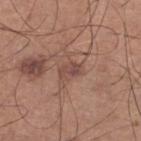Captured during whole-body skin photography for melanoma surveillance; the lesion was not biopsied. A male subject aged around 60. Located on the left lower leg. Measured at roughly 2.5 mm in maximum diameter. A 15 mm close-up extracted from a 3D total-body photography capture.